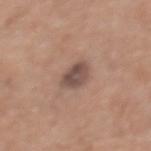Assessment: The lesion was tiled from a total-body skin photograph and was not biopsied. Background: This is a white-light tile. From the upper back. About 3 mm across. A female subject aged 58–62. A close-up tile cropped from a whole-body skin photograph, about 15 mm across.